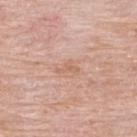Q: Was this lesion biopsied?
A: catalogued during a skin exam; not biopsied
Q: What is the imaging modality?
A: ~15 mm crop, total-body skin-cancer survey
Q: What are the patient's age and sex?
A: female, aged 63 to 67
Q: What did automated image analysis measure?
A: a shape eccentricity near 0.85 and two-axis asymmetry of about 0.6; an automated nevus-likeness rating near 0 out of 100 and a detector confidence of about 100 out of 100 that the crop contains a lesion
Q: What is the lesion's diameter?
A: ~2.5 mm (longest diameter)
Q: How was the tile lit?
A: white-light
Q: What is the anatomic site?
A: the upper back